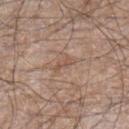Case summary:
• notes — imaged on a skin check; not biopsied
• imaging modality — 15 mm crop, total-body photography
• lighting — white-light
• body site — the right lower leg
• lesion size — ≈2.5 mm
• patient — male, aged 53–57